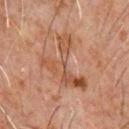Assessment:
The lesion was tiled from a total-body skin photograph and was not biopsied.
Image and clinical context:
The lesion is on the chest. A male subject, in their 60s. A 15 mm close-up tile from a total-body photography series done for melanoma screening. An algorithmic analysis of the crop reported a border-irregularity index near 10/10, internal color variation of about 7.5 on a 0–10 scale, and a peripheral color-asymmetry measure near 2. It also reported a classifier nevus-likeness of about 0/100 and a lesion-detection confidence of about 100/100.